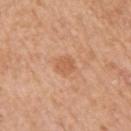notes: catalogued during a skin exam; not biopsied
tile lighting: white-light
patient: male, aged 53 to 57
automated metrics: a footprint of about 5 mm², a shape eccentricity near 0.45, and a symmetry-axis asymmetry near 0.2; an automated nevus-likeness rating near 30 out of 100 and a lesion-detection confidence of about 100/100
lesion size: ≈3 mm
site: the arm
image source: ~15 mm tile from a whole-body skin photo